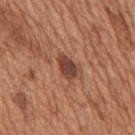Recorded during total-body skin imaging; not selected for excision or biopsy.
The subject is a male roughly 65 years of age.
Approximately 3 mm at its widest.
Cropped from a whole-body photographic skin survey; the tile spans about 15 mm.
Located on the back.